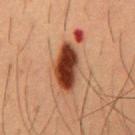Recorded during total-body skin imaging; not selected for excision or biopsy. The tile uses cross-polarized illumination. The lesion's longest dimension is about 6 mm. The patient is a male in their mid- to late 50s. A roughly 15 mm field-of-view crop from a total-body skin photograph. From the mid back.